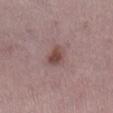* workup · imaged on a skin check; not biopsied
* lesion size · about 3 mm
* patient · female, aged 23 to 27
* image source · ~15 mm crop, total-body skin-cancer survey
* tile lighting · white-light
* site · the right lower leg